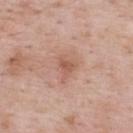Acquisition and patient details: Captured under white-light illumination. From the mid back. A lesion tile, about 15 mm wide, cut from a 3D total-body photograph. A male patient, aged approximately 55. Automated image analysis of the tile measured an area of roughly 2 mm², a shape eccentricity near 0.65, and two-axis asymmetry of about 0.2. It also reported a mean CIELAB color near L≈55 a*≈23 b*≈29, a lesion–skin lightness drop of about 9, and a lesion-to-skin contrast of about 6 (normalized; higher = more distinct). And it measured a border-irregularity rating of about 2/10 and radial color variation of about 0. The analysis additionally found a classifier nevus-likeness of about 0/100.